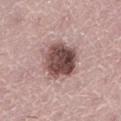  image:
    source: total-body photography crop
    field_of_view_mm: 15
  patient:
    sex: female
    age_approx: 40
  site: left lower leg